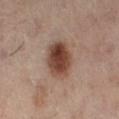biopsy status: catalogued during a skin exam; not biopsied | anatomic site: the left leg | acquisition: ~15 mm crop, total-body skin-cancer survey | patient: female, in their mid-50s.